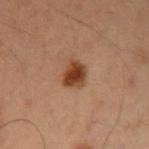Part of a total-body skin-imaging series; this lesion was reviewed on a skin check and was not flagged for biopsy. Located on the left upper arm. This is a cross-polarized tile. The patient is a male about 50 years old. A lesion tile, about 15 mm wide, cut from a 3D total-body photograph. Approximately 3 mm at its widest.